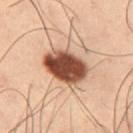Imaged during a routine full-body skin examination; the lesion was not biopsied and no histopathology is available. The tile uses cross-polarized illumination. A region of skin cropped from a whole-body photographic capture, roughly 15 mm wide. The subject is a male about 55 years old. From the leg. The recorded lesion diameter is about 4.5 mm.